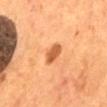Imaged during a routine full-body skin examination; the lesion was not biopsied and no histopathology is available. Located on the back. The recorded lesion diameter is about 3.5 mm. This is a cross-polarized tile. Automated image analysis of the tile measured a border-irregularity rating of about 2.5/10, a within-lesion color-variation index near 1.5/10, and peripheral color asymmetry of about 0.5. And it measured a classifier nevus-likeness of about 85/100 and a detector confidence of about 100 out of 100 that the crop contains a lesion. A roughly 15 mm field-of-view crop from a total-body skin photograph. The subject is a male aged 53 to 57.